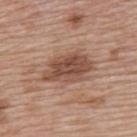Recorded during total-body skin imaging; not selected for excision or biopsy.
Located on the upper back.
Imaged with white-light lighting.
A 15 mm close-up extracted from a 3D total-body photography capture.
A female patient approximately 60 years of age.
Automated image analysis of the tile measured a lesion area of about 15 mm² and two-axis asymmetry of about 0.2. The analysis additionally found a lesion color around L≈49 a*≈20 b*≈28 in CIELAB, roughly 12 lightness units darker than nearby skin, and a normalized border contrast of about 8.5. The software also gave a peripheral color-asymmetry measure near 1.5.
The lesion's longest dimension is about 6 mm.